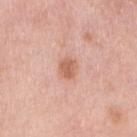  biopsy_status: not biopsied; imaged during a skin examination
  patient:
    sex: female
    age_approx: 65
  lesion_size:
    long_diameter_mm_approx: 2.5
  image:
    source: total-body photography crop
    field_of_view_mm: 15
  site: right upper arm
  lighting: white-light